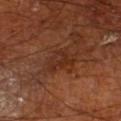Assessment:
No biopsy was performed on this lesion — it was imaged during a full skin examination and was not determined to be concerning.
Acquisition and patient details:
A region of skin cropped from a whole-body photographic capture, roughly 15 mm wide. Located on the left lower leg. A male patient, aged approximately 65.